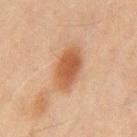Imaged during a routine full-body skin examination; the lesion was not biopsied and no histopathology is available.
The patient is a male aged around 45.
A 15 mm close-up extracted from a 3D total-body photography capture.
The tile uses cross-polarized illumination.
Located on the back.
Longest diameter approximately 4.5 mm.